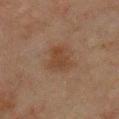<record>
<biopsy_status>not biopsied; imaged during a skin examination</biopsy_status>
<image>
  <source>total-body photography crop</source>
  <field_of_view_mm>15</field_of_view_mm>
</image>
<lighting>cross-polarized</lighting>
<automated_metrics>
  <area_mm2_approx>8.5</area_mm2_approx>
  <eccentricity>0.65</eccentricity>
  <shape_asymmetry>0.35</shape_asymmetry>
  <cielab_L>33</cielab_L>
  <cielab_a>15</cielab_a>
  <cielab_b>25</cielab_b>
  <vs_skin_darker_L>6.0</vs_skin_darker_L>
  <vs_skin_contrast_norm>6.5</vs_skin_contrast_norm>
</automated_metrics>
<patient>
  <sex>male</sex>
  <age_approx>65</age_approx>
</patient>
<site>upper back</site>
<lesion_size>
  <long_diameter_mm_approx>4.0</long_diameter_mm_approx>
</lesion_size>
</record>